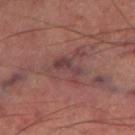The lesion was photographed on a routine skin check and not biopsied; there is no pathology result. A male subject, roughly 65 years of age. An algorithmic analysis of the crop reported an area of roughly 6 mm² and two-axis asymmetry of about 0.45. And it measured a mean CIELAB color near L≈40 a*≈21 b*≈17, about 7 CIELAB-L* units darker than the surrounding skin, and a normalized lesion–skin contrast near 7. A close-up tile cropped from a whole-body skin photograph, about 15 mm across. On the left lower leg. This is a cross-polarized tile.